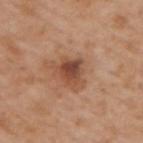<record>
  <biopsy_status>not biopsied; imaged during a skin examination</biopsy_status>
  <site>back</site>
  <automated_metrics>
    <area_mm2_approx>6.0</area_mm2_approx>
    <eccentricity>0.5</eccentricity>
    <shape_asymmetry>0.25</shape_asymmetry>
  </automated_metrics>
  <patient>
    <sex>male</sex>
    <age_approx>65</age_approx>
  </patient>
  <image>
    <source>total-body photography crop</source>
    <field_of_view_mm>15</field_of_view_mm>
  </image>
  <lighting>white-light</lighting>
</record>